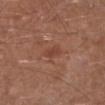biopsy status — no biopsy performed (imaged during a skin exam)
tile lighting — white-light
subject — male, approximately 65 years of age
image-analysis metrics — an area of roughly 2.5 mm², a shape eccentricity near 0.85, and a shape-asymmetry score of about 0.25 (0 = symmetric); a border-irregularity rating of about 2.5/10, a color-variation rating of about 0/10, and peripheral color asymmetry of about 0; a detector confidence of about 100 out of 100 that the crop contains a lesion
site — the right lower leg
image — total-body-photography crop, ~15 mm field of view